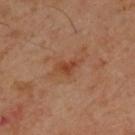* biopsy status · imaged on a skin check; not biopsied
* size · ~3 mm (longest diameter)
* image · 15 mm crop, total-body photography
* patient · male, approximately 45 years of age
* body site · the upper back
* tile lighting · cross-polarized